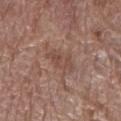| key | value |
|---|---|
| workup | imaged on a skin check; not biopsied |
| site | the left lower leg |
| subject | female, aged 78 to 82 |
| automated metrics | a footprint of about 6 mm², an eccentricity of roughly 0.85, and two-axis asymmetry of about 0.3; a color-variation rating of about 3/10 and radial color variation of about 1; a nevus-likeness score of about 0/100 and a detector confidence of about 100 out of 100 that the crop contains a lesion |
| illumination | white-light |
| image | 15 mm crop, total-body photography |